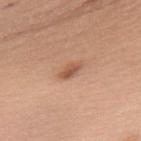The lesion was tiled from a total-body skin photograph and was not biopsied. The patient is a female aged approximately 60. This is a white-light tile. The lesion is on the back. A 15 mm close-up tile from a total-body photography series done for melanoma screening. An algorithmic analysis of the crop reported a symmetry-axis asymmetry near 0.3. The software also gave an average lesion color of about L≈55 a*≈22 b*≈32 (CIELAB), a lesion–skin lightness drop of about 11, and a normalized border contrast of about 7. The recorded lesion diameter is about 3 mm.